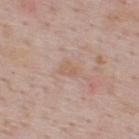Findings:
* workup: catalogued during a skin exam; not biopsied
* automated lesion analysis: a mean CIELAB color near L≈60 a*≈17 b*≈28 and about 5 CIELAB-L* units darker than the surrounding skin; a nevus-likeness score of about 0/100 and a detector confidence of about 100 out of 100 that the crop contains a lesion
* image source: ~15 mm tile from a whole-body skin photo
* site: the back
* lighting: white-light illumination
* subject: male, approximately 50 years of age